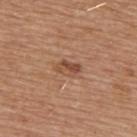Case summary:
- biopsy status: no biopsy performed (imaged during a skin exam)
- image: 15 mm crop, total-body photography
- location: the upper back
- subject: male, approximately 65 years of age